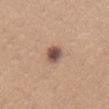Notes:
- notes — no biopsy performed (imaged during a skin exam)
- subject — female, approximately 55 years of age
- image — 15 mm crop, total-body photography
- automated lesion analysis — an automated nevus-likeness rating near 85 out of 100
- diameter — ~3 mm (longest diameter)
- lighting — white-light illumination
- site — the chest